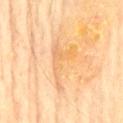Findings:
• follow-up — catalogued during a skin exam; not biopsied
• patient — male, aged 73–77
• imaging modality — ~15 mm crop, total-body skin-cancer survey
• image-analysis metrics — a lesion area of about 9.5 mm², an outline eccentricity of about 0.9 (0 = round, 1 = elongated), and a shape-asymmetry score of about 0.75 (0 = symmetric); a classifier nevus-likeness of about 0/100 and a detector confidence of about 70 out of 100 that the crop contains a lesion
• diameter — ~6.5 mm (longest diameter)
• lighting — cross-polarized
• body site — the mid back